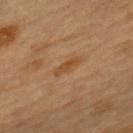• biopsy status · imaged on a skin check; not biopsied
• tile lighting · cross-polarized illumination
• diameter · ≈3 mm
• automated lesion analysis · a lesion area of about 3 mm² and an outline eccentricity of about 0.9 (0 = round, 1 = elongated); a border-irregularity rating of about 3.5/10, a within-lesion color-variation index near 0/10, and peripheral color asymmetry of about 0; a nevus-likeness score of about 20/100
• patient · female, about 60 years old
• acquisition · total-body-photography crop, ~15 mm field of view
• body site · the mid back